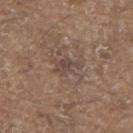No biopsy was performed on this lesion — it was imaged during a full skin examination and was not determined to be concerning. A male patient aged 78 to 82. The lesion's longest dimension is about 3.5 mm. Cropped from a total-body skin-imaging series; the visible field is about 15 mm. The lesion is on the upper back. The tile uses white-light illumination.